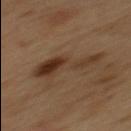Assessment: This lesion was catalogued during total-body skin photography and was not selected for biopsy.